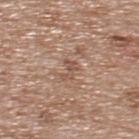| field | value |
|---|---|
| workup | catalogued during a skin exam; not biopsied |
| lesion size | ~2.5 mm (longest diameter) |
| location | the upper back |
| acquisition | 15 mm crop, total-body photography |
| patient | male, roughly 65 years of age |